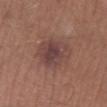Notes:
* biopsy status — catalogued during a skin exam; not biopsied
* size — ~4.5 mm (longest diameter)
* acquisition — ~15 mm crop, total-body skin-cancer survey
* anatomic site — the right lower leg
* patient — male, approximately 65 years of age